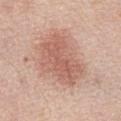Q: Was this lesion biopsied?
A: imaged on a skin check; not biopsied
Q: What lighting was used for the tile?
A: white-light
Q: What is the anatomic site?
A: the abdomen
Q: Automated lesion metrics?
A: an area of roughly 23 mm², a shape eccentricity near 0.75, and two-axis asymmetry of about 0.25; a nevus-likeness score of about 90/100 and lesion-presence confidence of about 100/100
Q: What are the patient's age and sex?
A: male, approximately 70 years of age
Q: What is the lesion's diameter?
A: about 7 mm
Q: What kind of image is this?
A: ~15 mm crop, total-body skin-cancer survey